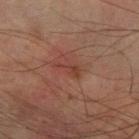Part of a total-body skin-imaging series; this lesion was reviewed on a skin check and was not flagged for biopsy.
Measured at roughly 3 mm in maximum diameter.
A 15 mm close-up extracted from a 3D total-body photography capture.
Located on the right forearm.
A male patient, in their mid-70s.